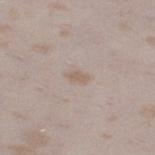Part of a total-body skin-imaging series; this lesion was reviewed on a skin check and was not flagged for biopsy. The subject is a female approximately 25 years of age. A 15 mm close-up tile from a total-body photography series done for melanoma screening. Located on the leg.